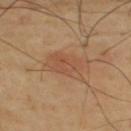No biopsy was performed on this lesion — it was imaged during a full skin examination and was not determined to be concerning. A region of skin cropped from a whole-body photographic capture, roughly 15 mm wide. The subject is a male roughly 65 years of age. Automated tile analysis of the lesion measured a mean CIELAB color near L≈49 a*≈21 b*≈32, roughly 7 lightness units darker than nearby skin, and a normalized border contrast of about 5. It also reported a nevus-likeness score of about 55/100. Located on the upper back.